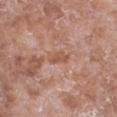This lesion was catalogued during total-body skin photography and was not selected for biopsy. On the chest. This image is a 15 mm lesion crop taken from a total-body photograph. The patient is a female in their mid-70s.